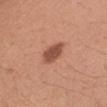Notes:
* biopsy status: imaged on a skin check; not biopsied
* site: the right upper arm
* subject: female, approximately 40 years of age
* image source: ~15 mm crop, total-body skin-cancer survey
* image-analysis metrics: a lesion area of about 6 mm², an eccentricity of roughly 0.75, and a shape-asymmetry score of about 0.2 (0 = symmetric)
* size: about 3.5 mm